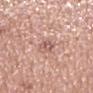Recorded during total-body skin imaging; not selected for excision or biopsy. Longest diameter approximately 3 mm. The patient is a male in their mid-60s. An algorithmic analysis of the crop reported an area of roughly 4 mm², an outline eccentricity of about 0.8 (0 = round, 1 = elongated), and a shape-asymmetry score of about 0.2 (0 = symmetric). The software also gave an average lesion color of about L≈58 a*≈23 b*≈25 (CIELAB), a lesion–skin lightness drop of about 10, and a normalized border contrast of about 6. A roughly 15 mm field-of-view crop from a total-body skin photograph. Located on the mid back. Captured under white-light illumination.